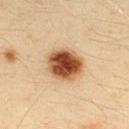workup=no biopsy performed (imaged during a skin exam) | size=≈4.5 mm | image=15 mm crop, total-body photography | TBP lesion metrics=a lesion color around L≈46 a*≈22 b*≈34 in CIELAB, about 20 CIELAB-L* units darker than the surrounding skin, and a normalized border contrast of about 13.5; a border-irregularity index near 1.5/10, internal color variation of about 7.5 on a 0–10 scale, and peripheral color asymmetry of about 2 | patient=male, aged 33 to 37 | body site=the upper back | illumination=cross-polarized illumination.